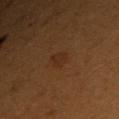Impression:
Imaged during a routine full-body skin examination; the lesion was not biopsied and no histopathology is available.
Image and clinical context:
Imaged with cross-polarized lighting. The lesion is located on the right upper arm. A 15 mm crop from a total-body photograph taken for skin-cancer surveillance. The subject is a male approximately 50 years of age.